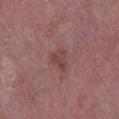Impression:
Part of a total-body skin-imaging series; this lesion was reviewed on a skin check and was not flagged for biopsy.
Clinical summary:
The subject is a female approximately 70 years of age. Measured at roughly 3 mm in maximum diameter. Automated image analysis of the tile measured an area of roughly 4.5 mm², a shape eccentricity near 0.65, and a shape-asymmetry score of about 0.4 (0 = symmetric). The analysis additionally found a lesion–skin lightness drop of about 7 and a normalized border contrast of about 6. And it measured an automated nevus-likeness rating near 0 out of 100 and a lesion-detection confidence of about 100/100. On the left lower leg. Captured under white-light illumination. A 15 mm crop from a total-body photograph taken for skin-cancer surveillance.